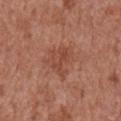Captured during whole-body skin photography for melanoma surveillance; the lesion was not biopsied. A male patient approximately 65 years of age. About 4 mm across. Automated image analysis of the tile measured an average lesion color of about L≈47 a*≈25 b*≈30 (CIELAB), a lesion–skin lightness drop of about 7, and a lesion-to-skin contrast of about 5.5 (normalized; higher = more distinct). It also reported a border-irregularity index near 4.5/10, a within-lesion color-variation index near 3/10, and radial color variation of about 1. And it measured a nevus-likeness score of about 0/100. From the arm. A 15 mm close-up tile from a total-body photography series done for melanoma screening.